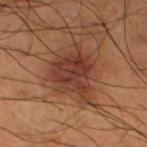notes = no biopsy performed (imaged during a skin exam) | acquisition = total-body-photography crop, ~15 mm field of view | patient = male, aged around 65 | site = the left thigh | illumination = cross-polarized.